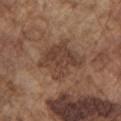The lesion was tiled from a total-body skin photograph and was not biopsied. The tile uses white-light illumination. On the left upper arm. Longest diameter approximately 5.5 mm. A region of skin cropped from a whole-body photographic capture, roughly 15 mm wide. A male subject, aged 73 to 77.